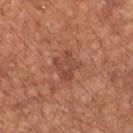workup — total-body-photography surveillance lesion; no biopsy | imaging modality — ~15 mm crop, total-body skin-cancer survey | location — the chest | patient — male, approximately 65 years of age.